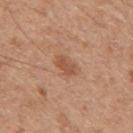Captured during whole-body skin photography for melanoma surveillance; the lesion was not biopsied. This image is a 15 mm lesion crop taken from a total-body photograph. The subject is a male in their mid- to late 40s. The lesion is located on the mid back. Captured under white-light illumination. The recorded lesion diameter is about 3 mm. The total-body-photography lesion software estimated a lesion area of about 4.5 mm² and a symmetry-axis asymmetry near 0.2. The software also gave a lesion color around L≈53 a*≈22 b*≈33 in CIELAB and a normalized border contrast of about 6.